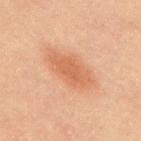| feature | finding |
|---|---|
| biopsy status | catalogued during a skin exam; not biopsied |
| automated lesion analysis | an outline eccentricity of about 0.9 (0 = round, 1 = elongated); internal color variation of about 2 on a 0–10 scale and peripheral color asymmetry of about 0.5; a nevus-likeness score of about 100/100 and a detector confidence of about 100 out of 100 that the crop contains a lesion |
| patient | female, aged 63 to 67 |
| tile lighting | cross-polarized illumination |
| anatomic site | the upper back |
| diameter | ~5.5 mm (longest diameter) |
| image | ~15 mm tile from a whole-body skin photo |